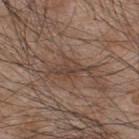The lesion was tiled from a total-body skin photograph and was not biopsied. On the upper back. A male subject, aged 43–47. Automated tile analysis of the lesion measured a lesion area of about 7 mm² and two-axis asymmetry of about 0.4. It also reported a lesion color around L≈42 a*≈15 b*≈24 in CIELAB, about 8 CIELAB-L* units darker than the surrounding skin, and a lesion-to-skin contrast of about 6.5 (normalized; higher = more distinct). A 15 mm crop from a total-body photograph taken for skin-cancer surveillance. The tile uses white-light illumination.